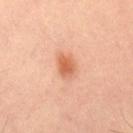Case summary:
– notes · catalogued during a skin exam; not biopsied
– diameter · ≈2.5 mm
– subject · male, aged approximately 55
– lighting · cross-polarized
– site · the leg
– image source · ~15 mm tile from a whole-body skin photo